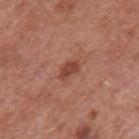| key | value |
|---|---|
| biopsy status | catalogued during a skin exam; not biopsied |
| location | the mid back |
| subject | male, aged 63 to 67 |
| image | total-body-photography crop, ~15 mm field of view |
| illumination | white-light |
| image-analysis metrics | a symmetry-axis asymmetry near 0.3; an average lesion color of about L≈44 a*≈26 b*≈29 (CIELAB) and about 10 CIELAB-L* units darker than the surrounding skin |